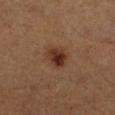{
  "biopsy_status": "not biopsied; imaged during a skin examination",
  "image": {
    "source": "total-body photography crop",
    "field_of_view_mm": 15
  },
  "lighting": "cross-polarized",
  "patient": {
    "sex": "male",
    "age_approx": 60
  },
  "site": "left lower leg"
}